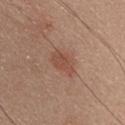Recorded during total-body skin imaging; not selected for excision or biopsy.
The lesion is located on the upper back.
Imaged with white-light lighting.
The patient is a female roughly 35 years of age.
About 3.5 mm across.
The total-body-photography lesion software estimated a border-irregularity index near 2.5/10, a within-lesion color-variation index near 2/10, and radial color variation of about 0.5.
A close-up tile cropped from a whole-body skin photograph, about 15 mm across.